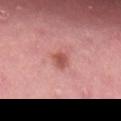biopsy_status: not biopsied; imaged during a skin examination
lighting: white-light
patient:
  sex: female
  age_approx: 50
lesion_size:
  long_diameter_mm_approx: 2.5
site: lower back
image:
  source: total-body photography crop
  field_of_view_mm: 15